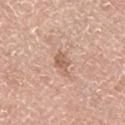lesion diameter — about 3 mm | subject — male, in their mid- to late 70s | imaging modality — ~15 mm tile from a whole-body skin photo | illumination — white-light | automated lesion analysis — an area of roughly 4 mm², an eccentricity of roughly 0.8, and two-axis asymmetry of about 0.45; a mean CIELAB color near L≈60 a*≈21 b*≈30, a lesion–skin lightness drop of about 10, and a lesion-to-skin contrast of about 6.5 (normalized; higher = more distinct); an automated nevus-likeness rating near 0 out of 100 | location — the right lower leg.